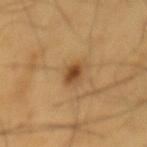Part of a total-body skin-imaging series; this lesion was reviewed on a skin check and was not flagged for biopsy.
This image is a 15 mm lesion crop taken from a total-body photograph.
Longest diameter approximately 2.5 mm.
A male patient, approximately 60 years of age.
The tile uses cross-polarized illumination.
On the mid back.